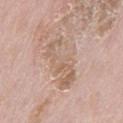Acquisition and patient details: Longest diameter approximately 7 mm. On the mid back. Imaged with white-light lighting. A male patient aged around 65. A 15 mm close-up extracted from a 3D total-body photography capture.